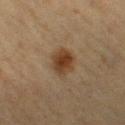| feature | finding |
|---|---|
| biopsy status | catalogued during a skin exam; not biopsied |
| lesion size | ≈3.5 mm |
| acquisition | total-body-photography crop, ~15 mm field of view |
| patient | female, roughly 55 years of age |
| illumination | cross-polarized |
| site | the left thigh |